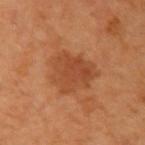| field | value |
|---|---|
| follow-up | no biopsy performed (imaged during a skin exam) |
| diameter | ≈5.5 mm |
| acquisition | ~15 mm tile from a whole-body skin photo |
| subject | male, about 60 years old |
| anatomic site | the left upper arm |
| tile lighting | cross-polarized illumination |